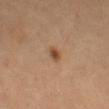biopsy status: no biopsy performed (imaged during a skin exam)
TBP lesion metrics: a lesion area of about 2.5 mm², an eccentricity of roughly 0.75, and a shape-asymmetry score of about 0.2 (0 = symmetric); a lesion–skin lightness drop of about 10; a border-irregularity index near 1.5/10 and a peripheral color-asymmetry measure near 1; a nevus-likeness score of about 95/100 and a lesion-detection confidence of about 100/100
location: the left thigh
illumination: cross-polarized illumination
imaging modality: 15 mm crop, total-body photography
patient: female, aged around 65
lesion diameter: ~2 mm (longest diameter)